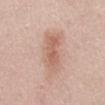Recorded during total-body skin imaging; not selected for excision or biopsy.